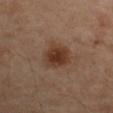Q: Was a biopsy performed?
A: imaged on a skin check; not biopsied
Q: What is the anatomic site?
A: the right upper arm
Q: What kind of image is this?
A: total-body-photography crop, ~15 mm field of view
Q: How was the tile lit?
A: cross-polarized illumination
Q: What are the patient's age and sex?
A: male, approximately 55 years of age
Q: What is the lesion's diameter?
A: about 4 mm